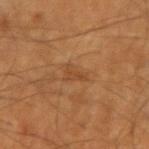biopsy_status: not biopsied; imaged during a skin examination
patient:
  sex: male
  age_approx: 65
site: left upper arm
image:
  source: total-body photography crop
  field_of_view_mm: 15
automated_metrics:
  cielab_L: 41
  cielab_a: 20
  cielab_b: 34
  vs_skin_darker_L: 6.0
  nevus_likeness_0_100: 0
  lesion_detection_confidence_0_100: 100